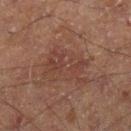  biopsy_status: not biopsied; imaged during a skin examination
  automated_metrics:
    vs_skin_darker_L: 5.0
    vs_skin_contrast_norm: 5.0
  patient:
    sex: male
    age_approx: 60
  site: left leg
  lighting: cross-polarized
  image:
    source: total-body photography crop
    field_of_view_mm: 15
  lesion_size:
    long_diameter_mm_approx: 6.0